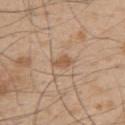biopsy_status: not biopsied; imaged during a skin examination
site: upper back
image:
  source: total-body photography crop
  field_of_view_mm: 15
patient:
  sex: male
  age_approx: 50
lighting: white-light